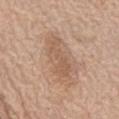Q: Is there a histopathology result?
A: imaged on a skin check; not biopsied
Q: Lesion location?
A: the abdomen
Q: How was this image acquired?
A: 15 mm crop, total-body photography
Q: Patient demographics?
A: male, in their mid-60s
Q: How large is the lesion?
A: ≈5 mm